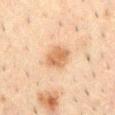notes — imaged on a skin check; not biopsied
image — ~15 mm tile from a whole-body skin photo
illumination — cross-polarized
subject — male, in their 50s
size — about 3.5 mm
image-analysis metrics — a lesion area of about 7 mm² and a shape eccentricity near 0.7; an average lesion color of about L≈51 a*≈17 b*≈31 (CIELAB) and a lesion-to-skin contrast of about 7.5 (normalized; higher = more distinct)
site — the abdomen